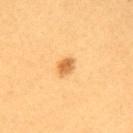• workup — catalogued during a skin exam; not biopsied
• image source — ~15 mm tile from a whole-body skin photo
• site — the back
• automated lesion analysis — a lesion area of about 4 mm², an eccentricity of roughly 0.7, and two-axis asymmetry of about 0.2; an average lesion color of about L≈66 a*≈24 b*≈49 (CIELAB) and about 13 CIELAB-L* units darker than the surrounding skin
• patient — female, approximately 25 years of age
• lighting — cross-polarized